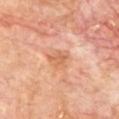Q: Where on the body is the lesion?
A: the front of the torso
Q: How was the tile lit?
A: cross-polarized illumination
Q: What are the patient's age and sex?
A: male, about 70 years old
Q: How large is the lesion?
A: ≈3 mm
Q: What is the imaging modality?
A: ~15 mm crop, total-body skin-cancer survey
Q: Automated lesion metrics?
A: a border-irregularity index near 3/10, internal color variation of about 2.5 on a 0–10 scale, and a peripheral color-asymmetry measure near 1